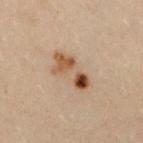The lesion was photographed on a routine skin check and not biopsied; there is no pathology result.
A female patient in their 30s.
A roughly 15 mm field-of-view crop from a total-body skin photograph.
The lesion is located on the chest.
Captured under cross-polarized illumination.